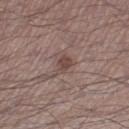Q: Was a biopsy performed?
A: imaged on a skin check; not biopsied
Q: How was this image acquired?
A: ~15 mm crop, total-body skin-cancer survey
Q: Lesion location?
A: the leg
Q: Who is the patient?
A: male, in their 70s